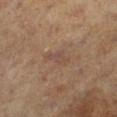biopsy status: no biopsy performed (imaged during a skin exam)
image: total-body-photography crop, ~15 mm field of view
site: the left leg
illumination: cross-polarized
TBP lesion metrics: a lesion-detection confidence of about 100/100
diameter: about 3.5 mm
subject: female, approximately 65 years of age